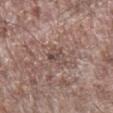No biopsy was performed on this lesion — it was imaged during a full skin examination and was not determined to be concerning. From the right lower leg. A male patient approximately 70 years of age. Cropped from a whole-body photographic skin survey; the tile spans about 15 mm. Automated tile analysis of the lesion measured an eccentricity of roughly 0.8 and a shape-asymmetry score of about 0.3 (0 = symmetric). The analysis additionally found a mean CIELAB color near L≈48 a*≈17 b*≈20 and about 7 CIELAB-L* units darker than the surrounding skin. Approximately 3 mm at its widest.